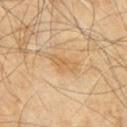Captured during whole-body skin photography for melanoma surveillance; the lesion was not biopsied. Measured at roughly 3.5 mm in maximum diameter. On the chest. A close-up tile cropped from a whole-body skin photograph, about 15 mm across. A male patient aged 58 to 62.